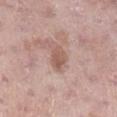{
  "biopsy_status": "not biopsied; imaged during a skin examination",
  "site": "right lower leg",
  "patient": {
    "sex": "female",
    "age_approx": 70
  },
  "image": {
    "source": "total-body photography crop",
    "field_of_view_mm": 15
  }
}